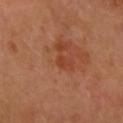Findings:
* biopsy status · catalogued during a skin exam; not biopsied